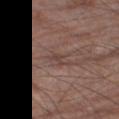Background:
The lesion's longest dimension is about 3 mm. This is a white-light tile. A 15 mm close-up tile from a total-body photography series done for melanoma screening. The total-body-photography lesion software estimated an area of roughly 3.5 mm² and an outline eccentricity of about 0.9 (0 = round, 1 = elongated). And it measured an average lesion color of about L≈43 a*≈17 b*≈22 (CIELAB), a lesion–skin lightness drop of about 7, and a normalized lesion–skin contrast near 5.5. A male subject aged approximately 80. On the right thigh.